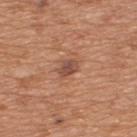Impression:
The lesion was photographed on a routine skin check and not biopsied; there is no pathology result.
Acquisition and patient details:
A male subject, aged approximately 65. Cropped from a total-body skin-imaging series; the visible field is about 15 mm. From the upper back.